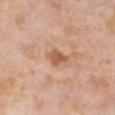Imaged during a routine full-body skin examination; the lesion was not biopsied and no histopathology is available. A region of skin cropped from a whole-body photographic capture, roughly 15 mm wide. The recorded lesion diameter is about 3 mm. Automated image analysis of the tile measured a border-irregularity rating of about 3/10, a color-variation rating of about 2/10, and peripheral color asymmetry of about 0.5. Located on the right lower leg. A female patient, roughly 55 years of age.